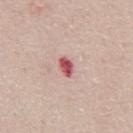The lesion was photographed on a routine skin check and not biopsied; there is no pathology result. A male subject aged 63–67. From the abdomen. A close-up tile cropped from a whole-body skin photograph, about 15 mm across.